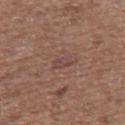The lesion was photographed on a routine skin check and not biopsied; there is no pathology result.
The tile uses white-light illumination.
The lesion's longest dimension is about 2.5 mm.
From the upper back.
A 15 mm close-up extracted from a 3D total-body photography capture.
The patient is a male aged approximately 60.